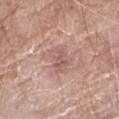diameter: about 3 mm | acquisition: ~15 mm tile from a whole-body skin photo | lighting: white-light | location: the right forearm | image-analysis metrics: a mean CIELAB color near L≈57 a*≈22 b*≈23, a lesion–skin lightness drop of about 8, and a lesion-to-skin contrast of about 5.5 (normalized; higher = more distinct); border irregularity of about 3 on a 0–10 scale and internal color variation of about 4 on a 0–10 scale; a classifier nevus-likeness of about 0/100 | patient: male, roughly 80 years of age.